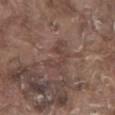The lesion was tiled from a total-body skin photograph and was not biopsied. From the front of the torso. Imaged with white-light lighting. A region of skin cropped from a whole-body photographic capture, roughly 15 mm wide. The patient is a male roughly 80 years of age. Measured at roughly 3.5 mm in maximum diameter.